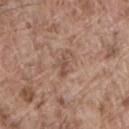biopsy_status: not biopsied; imaged during a skin examination
automated_metrics:
  border_irregularity_0_10: 5.0
  color_variation_0_10: 1.0
  peripheral_color_asymmetry: 0.0
image:
  source: total-body photography crop
  field_of_view_mm: 15
site: lower back
lesion_size:
  long_diameter_mm_approx: 3.0
patient:
  sex: male
  age_approx: 80
lighting: white-light